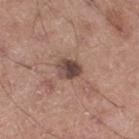notes: catalogued during a skin exam; not biopsied | automated metrics: a footprint of about 5.5 mm² and an eccentricity of roughly 0.6; a mean CIELAB color near L≈45 a*≈18 b*≈21 and a lesion–skin lightness drop of about 13; internal color variation of about 6 on a 0–10 scale and a peripheral color-asymmetry measure near 2.5 | lesion size: about 3 mm | subject: male, approximately 55 years of age | acquisition: ~15 mm tile from a whole-body skin photo | location: the right thigh.